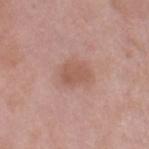Clinical impression: Imaged during a routine full-body skin examination; the lesion was not biopsied and no histopathology is available. Background: The patient is a female aged approximately 70. A close-up tile cropped from a whole-body skin photograph, about 15 mm across. On the leg.